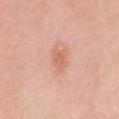biopsy_status: not biopsied; imaged during a skin examination
lesion_size:
  long_diameter_mm_approx: 2.5
lighting: white-light
site: right upper arm
patient:
  sex: female
  age_approx: 25
automated_metrics:
  area_mm2_approx: 4.0
  shape_asymmetry: 0.25
  cielab_L: 64
  cielab_a: 26
  cielab_b: 32
  border_irregularity_0_10: 3.0
  peripheral_color_asymmetry: 0.5
image:
  source: total-body photography crop
  field_of_view_mm: 15